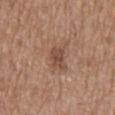Clinical impression:
No biopsy was performed on this lesion — it was imaged during a full skin examination and was not determined to be concerning.
Context:
The patient is a male approximately 70 years of age. Imaged with white-light lighting. A 15 mm crop from a total-body photograph taken for skin-cancer surveillance. An algorithmic analysis of the crop reported an average lesion color of about L≈48 a*≈21 b*≈27 (CIELAB), roughly 9 lightness units darker than nearby skin, and a lesion-to-skin contrast of about 7 (normalized; higher = more distinct). And it measured a border-irregularity rating of about 3.5/10 and peripheral color asymmetry of about 1. It also reported a classifier nevus-likeness of about 20/100 and a detector confidence of about 100 out of 100 that the crop contains a lesion. The lesion is located on the mid back. About 3.5 mm across.